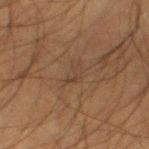follow-up: imaged on a skin check; not biopsied
site: the left thigh
acquisition: ~15 mm crop, total-body skin-cancer survey
lighting: cross-polarized
subject: male, in their mid- to late 30s